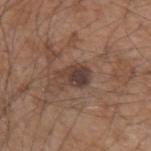Captured during whole-body skin photography for melanoma surveillance; the lesion was not biopsied.
A lesion tile, about 15 mm wide, cut from a 3D total-body photograph.
This is a white-light tile.
The recorded lesion diameter is about 3.5 mm.
From the left forearm.
A male patient in their mid-50s.